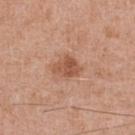Part of a total-body skin-imaging series; this lesion was reviewed on a skin check and was not flagged for biopsy. Imaged with white-light lighting. About 3.5 mm across. The lesion-visualizer software estimated a lesion color around L≈53 a*≈24 b*≈33 in CIELAB, about 10 CIELAB-L* units darker than the surrounding skin, and a normalized lesion–skin contrast near 7.5. And it measured an automated nevus-likeness rating near 60 out of 100 and a detector confidence of about 100 out of 100 that the crop contains a lesion. Located on the front of the torso. Cropped from a whole-body photographic skin survey; the tile spans about 15 mm. A male subject, in their mid- to late 50s.